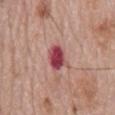Acquisition and patient details:
Imaged with white-light lighting. The patient is a male aged 78 to 82. A 15 mm close-up tile from a total-body photography series done for melanoma screening. Approximately 4 mm at its widest. The lesion is located on the mid back. Automated image analysis of the tile measured a lesion area of about 7 mm², a shape eccentricity near 0.8, and a symmetry-axis asymmetry near 0.25. It also reported a normalized border contrast of about 12. And it measured a border-irregularity rating of about 2.5/10, a within-lesion color-variation index near 4/10, and a peripheral color-asymmetry measure near 1.5. And it measured an automated nevus-likeness rating near 0 out of 100 and lesion-presence confidence of about 100/100.